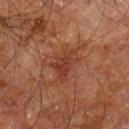Q: What lighting was used for the tile?
A: cross-polarized illumination
Q: How was this image acquired?
A: ~15 mm crop, total-body skin-cancer survey
Q: Who is the patient?
A: male, about 60 years old
Q: Lesion location?
A: the right leg